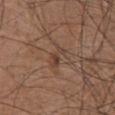| key | value |
|---|---|
| subject | male, about 75 years old |
| size | about 3 mm |
| site | the chest |
| imaging modality | ~15 mm tile from a whole-body skin photo |